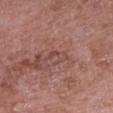Q: Is there a histopathology result?
A: no biopsy performed (imaged during a skin exam)
Q: Illumination type?
A: white-light
Q: What is the imaging modality?
A: ~15 mm crop, total-body skin-cancer survey
Q: Lesion size?
A: ~3.5 mm (longest diameter)
Q: What are the patient's age and sex?
A: female, aged 68–72
Q: Lesion location?
A: the right upper arm